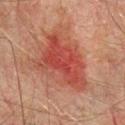{"automated_metrics": {"cielab_L": 40, "cielab_a": 27, "cielab_b": 26, "vs_skin_darker_L": 9.0, "vs_skin_contrast_norm": 7.5, "border_irregularity_0_10": 4.0, "color_variation_0_10": 6.5, "peripheral_color_asymmetry": 2.0, "nevus_likeness_0_100": 20, "lesion_detection_confidence_0_100": 100}, "site": "chest", "lesion_size": {"long_diameter_mm_approx": 7.5}, "image": {"source": "total-body photography crop", "field_of_view_mm": 15}, "patient": {"sex": "male", "age_approx": 75}}